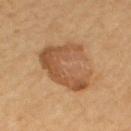Clinical impression:
No biopsy was performed on this lesion — it was imaged during a full skin examination and was not determined to be concerning.
Background:
Longest diameter approximately 6 mm. A female patient aged approximately 65. The total-body-photography lesion software estimated a border-irregularity rating of about 6.5/10, internal color variation of about 5 on a 0–10 scale, and peripheral color asymmetry of about 2. The software also gave a classifier nevus-likeness of about 60/100 and lesion-presence confidence of about 100/100. Imaged with cross-polarized lighting. A close-up tile cropped from a whole-body skin photograph, about 15 mm across. The lesion is on the left upper arm.